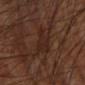Case summary:
* follow-up — catalogued during a skin exam; not biopsied
* body site — the right lower leg
* automated metrics — an outline eccentricity of about 0.75 (0 = round, 1 = elongated) and two-axis asymmetry of about 0.55; a mean CIELAB color near L≈23 a*≈18 b*≈22, about 5 CIELAB-L* units darker than the surrounding skin, and a lesion-to-skin contrast of about 5.5 (normalized; higher = more distinct); a classifier nevus-likeness of about 0/100 and a lesion-detection confidence of about 85/100
* lesion size — ≈4 mm
* image source — 15 mm crop, total-body photography
* patient — male, aged 58–62
* tile lighting — cross-polarized illumination